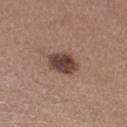Notes:
* biopsy status — no biopsy performed (imaged during a skin exam)
* body site — the right thigh
* image source — ~15 mm tile from a whole-body skin photo
* tile lighting — white-light illumination
* patient — female, about 35 years old